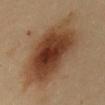The lesion was tiled from a total-body skin photograph and was not biopsied.
On the abdomen.
Approximately 11 mm at its widest.
The total-body-photography lesion software estimated an area of roughly 46 mm² and a symmetry-axis asymmetry near 0.2. The software also gave a lesion color around L≈43 a*≈20 b*≈32 in CIELAB, a lesion–skin lightness drop of about 14, and a normalized border contrast of about 11. It also reported a nevus-likeness score of about 100/100 and a lesion-detection confidence of about 100/100.
The subject is a female aged 48 to 52.
Imaged with cross-polarized lighting.
A roughly 15 mm field-of-view crop from a total-body skin photograph.